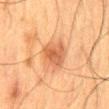{
  "biopsy_status": "not biopsied; imaged during a skin examination",
  "image": {
    "source": "total-body photography crop",
    "field_of_view_mm": 15
  },
  "automated_metrics": {
    "area_mm2_approx": 9.5,
    "eccentricity": 0.65,
    "shape_asymmetry": 0.25,
    "border_irregularity_0_10": 2.5,
    "peripheral_color_asymmetry": 1.0
  },
  "lesion_size": {
    "long_diameter_mm_approx": 4.0
  },
  "site": "mid back",
  "patient": {
    "sex": "male",
    "age_approx": 60
  }
}